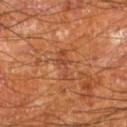| key | value |
|---|---|
| body site | the leg |
| lesion size | ≈4 mm |
| automated metrics | an automated nevus-likeness rating near 0 out of 100 and a lesion-detection confidence of about 85/100 |
| acquisition | ~15 mm tile from a whole-body skin photo |
| subject | male, approximately 65 years of age |